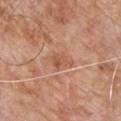Part of a total-body skin-imaging series; this lesion was reviewed on a skin check and was not flagged for biopsy.
A 15 mm crop from a total-body photograph taken for skin-cancer surveillance.
The patient is a male approximately 80 years of age.
Approximately 3 mm at its widest.
From the chest.
This is a white-light tile.
The total-body-photography lesion software estimated two-axis asymmetry of about 0.35. And it measured an average lesion color of about L≈55 a*≈25 b*≈34 (CIELAB), a lesion–skin lightness drop of about 8, and a normalized lesion–skin contrast near 6. The analysis additionally found an automated nevus-likeness rating near 5 out of 100 and a detector confidence of about 100 out of 100 that the crop contains a lesion.